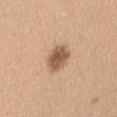The lesion was tiled from a total-body skin photograph and was not biopsied.
The lesion's longest dimension is about 3 mm.
A lesion tile, about 15 mm wide, cut from a 3D total-body photograph.
Imaged with white-light lighting.
A female patient, aged 28 to 32.
The lesion is on the right upper arm.
An algorithmic analysis of the crop reported a mean CIELAB color near L≈57 a*≈19 b*≈31, roughly 14 lightness units darker than nearby skin, and a normalized border contrast of about 9. The software also gave a border-irregularity rating of about 1.5/10, a color-variation rating of about 4/10, and peripheral color asymmetry of about 1. The analysis additionally found a nevus-likeness score of about 85/100 and a detector confidence of about 100 out of 100 that the crop contains a lesion.